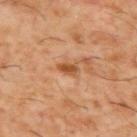This lesion was catalogued during total-body skin photography and was not selected for biopsy. Approximately 2.5 mm at its widest. This image is a 15 mm lesion crop taken from a total-body photograph. The subject is a male aged 58 to 62. The lesion is on the back.